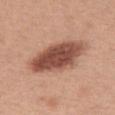notes: imaged on a skin check; not biopsied | lesion diameter: ~7 mm (longest diameter) | patient: female, aged approximately 55 | tile lighting: white-light illumination | automated lesion analysis: a mean CIELAB color near L≈50 a*≈23 b*≈28, about 16 CIELAB-L* units darker than the surrounding skin, and a lesion-to-skin contrast of about 11 (normalized; higher = more distinct); a within-lesion color-variation index near 5/10 and radial color variation of about 1.5; a classifier nevus-likeness of about 75/100 | imaging modality: 15 mm crop, total-body photography | location: the leg.